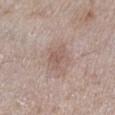| key | value |
|---|---|
| workup | no biopsy performed (imaged during a skin exam) |
| illumination | white-light |
| subject | female, aged 58–62 |
| image | total-body-photography crop, ~15 mm field of view |
| lesion diameter | ≈3.5 mm |
| anatomic site | the left lower leg |
| automated lesion analysis | a footprint of about 6 mm² and two-axis asymmetry of about 0.3; a lesion color around L≈57 a*≈17 b*≈23 in CIELAB and a normalized lesion–skin contrast near 5.5; a border-irregularity rating of about 3.5/10; a lesion-detection confidence of about 100/100 |